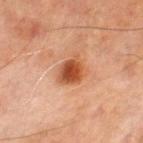Q: Is there a histopathology result?
A: imaged on a skin check; not biopsied
Q: What are the patient's age and sex?
A: male, aged around 70
Q: What did automated image analysis measure?
A: a footprint of about 7 mm², a shape eccentricity near 0.55, and a symmetry-axis asymmetry near 0.2
Q: What kind of image is this?
A: ~15 mm crop, total-body skin-cancer survey
Q: How was the tile lit?
A: cross-polarized
Q: Lesion size?
A: ~3.5 mm (longest diameter)
Q: Where on the body is the lesion?
A: the leg